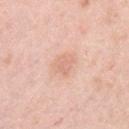The lesion was photographed on a routine skin check and not biopsied; there is no pathology result. A female subject aged approximately 65. This is a white-light tile. Cropped from a total-body skin-imaging series; the visible field is about 15 mm. The lesion is located on the left upper arm.